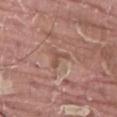Automated image analysis of the tile measured about 8 CIELAB-L* units darker than the surrounding skin and a normalized border contrast of about 6. It also reported a color-variation rating of about 0/10 and peripheral color asymmetry of about 0.
The lesion's longest dimension is about 2.5 mm.
From the left thigh.
The subject is a male approximately 40 years of age.
This is a white-light tile.
A close-up tile cropped from a whole-body skin photograph, about 15 mm across.